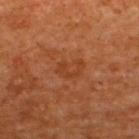Assessment:
No biopsy was performed on this lesion — it was imaged during a full skin examination and was not determined to be concerning.
Clinical summary:
Located on the upper back. Imaged with cross-polarized lighting. A 15 mm close-up tile from a total-body photography series done for melanoma screening. The patient is a male roughly 65 years of age.